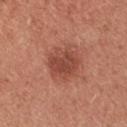  site: chest
  patient:
    sex: female
    age_approx: 35
  automated_metrics:
    area_mm2_approx: 11.0
    eccentricity: 0.6
    shape_asymmetry: 0.15
    cielab_L: 47
    cielab_a: 27
    cielab_b: 29
    vs_skin_darker_L: 10.0
    vs_skin_contrast_norm: 7.5
    nevus_likeness_0_100: 55
    lesion_detection_confidence_0_100: 100
  lighting: white-light
  lesion_size:
    long_diameter_mm_approx: 4.0
  image:
    source: total-body photography crop
    field_of_view_mm: 15